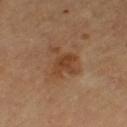subject: female, aged around 60
body site: the left thigh
automated lesion analysis: a lesion color around L≈44 a*≈21 b*≈33 in CIELAB, roughly 8 lightness units darker than nearby skin, and a lesion-to-skin contrast of about 7 (normalized; higher = more distinct); a border-irregularity index near 6/10 and a peripheral color-asymmetry measure near 1
imaging modality: ~15 mm crop, total-body skin-cancer survey